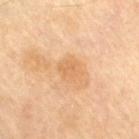Assessment:
Recorded during total-body skin imaging; not selected for excision or biopsy.
Image and clinical context:
The recorded lesion diameter is about 2.5 mm. A male subject approximately 70 years of age. Imaged with cross-polarized lighting. The lesion is on the right thigh. Cropped from a total-body skin-imaging series; the visible field is about 15 mm.